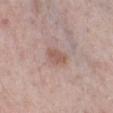The lesion was tiled from a total-body skin photograph and was not biopsied. The lesion's longest dimension is about 3 mm. The lesion is located on the right lower leg. Imaged with white-light lighting. A male patient aged 58 to 62. A 15 mm crop from a total-body photograph taken for skin-cancer surveillance.